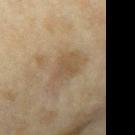Impression: Recorded during total-body skin imaging; not selected for excision or biopsy. Context: From the arm. Measured at roughly 3.5 mm in maximum diameter. Cropped from a whole-body photographic skin survey; the tile spans about 15 mm. Captured under cross-polarized illumination. The patient is a female in their 60s.